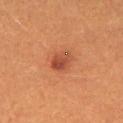biopsy status: total-body-photography surveillance lesion; no biopsy | anatomic site: the leg | lesion size: ~3 mm (longest diameter) | image: ~15 mm tile from a whole-body skin photo | subject: female, about 30 years old | tile lighting: cross-polarized.